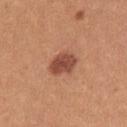biopsy status — total-body-photography surveillance lesion; no biopsy | tile lighting — white-light | subject — female, in their mid-20s | site — the left upper arm | image source — total-body-photography crop, ~15 mm field of view.